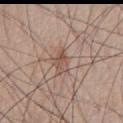Impression: Part of a total-body skin-imaging series; this lesion was reviewed on a skin check and was not flagged for biopsy. Image and clinical context: Located on the front of the torso. A male subject, aged around 80. Cropped from a whole-body photographic skin survey; the tile spans about 15 mm.